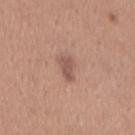{
  "biopsy_status": "not biopsied; imaged during a skin examination",
  "image": {
    "source": "total-body photography crop",
    "field_of_view_mm": 15
  },
  "patient": {
    "sex": "female",
    "age_approx": 30
  },
  "lighting": "white-light",
  "lesion_size": {
    "long_diameter_mm_approx": 3.0
  },
  "site": "lower back"
}